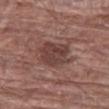Captured during whole-body skin photography for melanoma surveillance; the lesion was not biopsied. A 15 mm close-up extracted from a 3D total-body photography capture. Longest diameter approximately 4 mm. The subject is a male aged 68 to 72. The lesion is located on the left thigh.